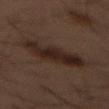Q: Was this lesion biopsied?
A: no biopsy performed (imaged during a skin exam)
Q: Illumination type?
A: cross-polarized
Q: What is the imaging modality?
A: total-body-photography crop, ~15 mm field of view
Q: What are the patient's age and sex?
A: male, about 55 years old
Q: What is the anatomic site?
A: the chest
Q: How large is the lesion?
A: ~6.5 mm (longest diameter)